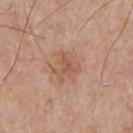Q: Is there a histopathology result?
A: total-body-photography surveillance lesion; no biopsy
Q: Patient demographics?
A: male, approximately 55 years of age
Q: Lesion location?
A: the upper back
Q: How was this image acquired?
A: 15 mm crop, total-body photography
Q: How was the tile lit?
A: white-light illumination